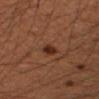Part of a total-body skin-imaging series; this lesion was reviewed on a skin check and was not flagged for biopsy. A lesion tile, about 15 mm wide, cut from a 3D total-body photograph. On the left forearm. A male subject about 50 years old. Longest diameter approximately 2 mm. This is a cross-polarized tile.